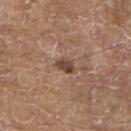Impression:
No biopsy was performed on this lesion — it was imaged during a full skin examination and was not determined to be concerning.
Image and clinical context:
Cropped from a total-body skin-imaging series; the visible field is about 15 mm. A male subject aged 78–82. From the back.